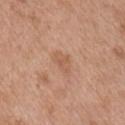image source=total-body-photography crop, ~15 mm field of view | illumination=white-light | site=the right upper arm | patient=male, aged around 55 | lesion diameter=about 3 mm.